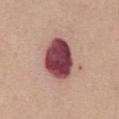<case>
<biopsy_status>not biopsied; imaged during a skin examination</biopsy_status>
<automated_metrics>
  <cielab_L>43</cielab_L>
  <cielab_a>29</cielab_a>
  <cielab_b>18</cielab_b>
  <vs_skin_darker_L>24.0</vs_skin_darker_L>
  <vs_skin_contrast_norm>16.5</vs_skin_contrast_norm>
  <border_irregularity_0_10>1.5</border_irregularity_0_10>
  <color_variation_0_10>6.0</color_variation_0_10>
  <peripheral_color_asymmetry>2.0</peripheral_color_asymmetry>
  <nevus_likeness_0_100>40</nevus_likeness_0_100>
  <lesion_detection_confidence_0_100>100</lesion_detection_confidence_0_100>
</automated_metrics>
<image>
  <source>total-body photography crop</source>
  <field_of_view_mm>15</field_of_view_mm>
</image>
<lighting>white-light</lighting>
<patient>
  <sex>female</sex>
  <age_approx>65</age_approx>
</patient>
<site>front of the torso</site>
</case>